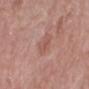follow-up=no biopsy performed (imaged during a skin exam) | patient=female, about 70 years old | diameter=≈3 mm | lighting=white-light | site=the right lower leg | imaging modality=~15 mm tile from a whole-body skin photo.